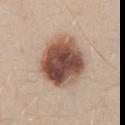<record>
  <biopsy_status>not biopsied; imaged during a skin examination</biopsy_status>
  <lighting>white-light</lighting>
  <lesion_size>
    <long_diameter_mm_approx>7.0</long_diameter_mm_approx>
  </lesion_size>
  <site>abdomen</site>
  <patient>
    <sex>male</sex>
    <age_approx>30</age_approx>
  </patient>
  <image>
    <source>total-body photography crop</source>
    <field_of_view_mm>15</field_of_view_mm>
  </image>
</record>